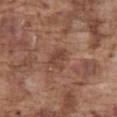acquisition: ~15 mm crop, total-body skin-cancer survey | image-analysis metrics: a mean CIELAB color near L≈43 a*≈21 b*≈27, a lesion–skin lightness drop of about 7, and a lesion-to-skin contrast of about 6 (normalized; higher = more distinct); internal color variation of about 3 on a 0–10 scale and radial color variation of about 1; an automated nevus-likeness rating near 0 out of 100 and a lesion-detection confidence of about 100/100 | subject: male, aged around 75 | anatomic site: the abdomen.